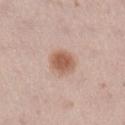| field | value |
|---|---|
| notes | catalogued during a skin exam; not biopsied |
| anatomic site | the right lower leg |
| subject | female, roughly 20 years of age |
| tile lighting | white-light illumination |
| lesion diameter | ≈3.5 mm |
| image | ~15 mm crop, total-body skin-cancer survey |